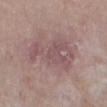notes: catalogued during a skin exam; not biopsied
body site: the left leg
image source: ~15 mm crop, total-body skin-cancer survey
illumination: white-light illumination
subject: female, aged 63–67